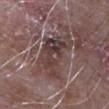Assessment:
Captured during whole-body skin photography for melanoma surveillance; the lesion was not biopsied.
Clinical summary:
Longest diameter approximately 7 mm. The patient is a male approximately 65 years of age. Located on the left upper arm. Imaged with white-light lighting. Cropped from a total-body skin-imaging series; the visible field is about 15 mm.